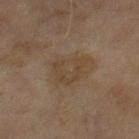follow-up: no biopsy performed (imaged during a skin exam) | site: the right thigh | acquisition: 15 mm crop, total-body photography | patient: female, aged 58–62.